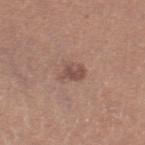Q: What are the patient's age and sex?
A: female, aged around 40
Q: What is the lesion's diameter?
A: ~3 mm (longest diameter)
Q: What is the anatomic site?
A: the right thigh
Q: What kind of image is this?
A: ~15 mm tile from a whole-body skin photo